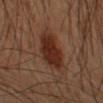follow-up: total-body-photography surveillance lesion; no biopsy
lighting: cross-polarized
subject: male, aged 48 to 52
image: 15 mm crop, total-body photography
location: the left forearm
lesion size: ~5 mm (longest diameter)